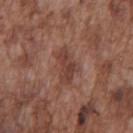Context: The subject is a male aged 73–77. The recorded lesion diameter is about 4 mm. The total-body-photography lesion software estimated a lesion area of about 7 mm² and an eccentricity of roughly 0.85. The software also gave a lesion color around L≈41 a*≈22 b*≈24 in CIELAB, a lesion–skin lightness drop of about 8, and a normalized border contrast of about 7. The tile uses white-light illumination. A lesion tile, about 15 mm wide, cut from a 3D total-body photograph. From the chest.